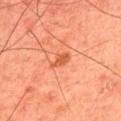biopsy_status: not biopsied; imaged during a skin examination
image:
  source: total-body photography crop
  field_of_view_mm: 15
patient:
  sex: male
  age_approx: 45
site: upper back